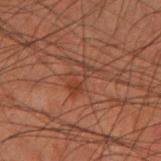notes: catalogued during a skin exam; not biopsied
automated lesion analysis: an area of roughly 4.5 mm²; a mean CIELAB color near L≈30 a*≈19 b*≈24, about 5 CIELAB-L* units darker than the surrounding skin, and a normalized border contrast of about 5; a border-irregularity rating of about 5.5/10 and peripheral color asymmetry of about 0.5
tile lighting: cross-polarized
image source: 15 mm crop, total-body photography
subject: male, aged around 60
site: the left forearm